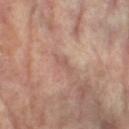Context:
This image is a 15 mm lesion crop taken from a total-body photograph. The lesion is located on the left thigh. The total-body-photography lesion software estimated a lesion area of about 3 mm², an outline eccentricity of about 0.9 (0 = round, 1 = elongated), and two-axis asymmetry of about 0.35. And it measured a border-irregularity rating of about 4/10 and peripheral color asymmetry of about 0. The analysis additionally found an automated nevus-likeness rating near 0 out of 100 and lesion-presence confidence of about 60/100. Longest diameter approximately 3 mm. A female patient, in their mid-70s.